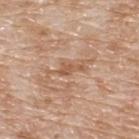Part of a total-body skin-imaging series; this lesion was reviewed on a skin check and was not flagged for biopsy.
About 3 mm across.
A male patient roughly 80 years of age.
The lesion is on the upper back.
An algorithmic analysis of the crop reported a footprint of about 3 mm², an eccentricity of roughly 0.9, and a symmetry-axis asymmetry near 0.6. The analysis additionally found internal color variation of about 0 on a 0–10 scale and peripheral color asymmetry of about 0.
Cropped from a total-body skin-imaging series; the visible field is about 15 mm.